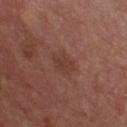Assessment: Captured during whole-body skin photography for melanoma surveillance; the lesion was not biopsied. Image and clinical context: The subject is a male in their mid-50s. From the front of the torso. Cropped from a total-body skin-imaging series; the visible field is about 15 mm.